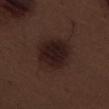Notes:
– workup · no biopsy performed (imaged during a skin exam)
– imaging modality · 15 mm crop, total-body photography
– site · the left thigh
– lesion size · about 5 mm
– automated metrics · a border-irregularity index near 1.5/10, a within-lesion color-variation index near 4/10, and radial color variation of about 1; an automated nevus-likeness rating near 85 out of 100 and lesion-presence confidence of about 100/100
– patient · male, aged 68 to 72
– illumination · white-light illumination